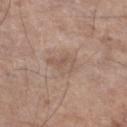{
  "biopsy_status": "not biopsied; imaged during a skin examination",
  "automated_metrics": {
    "cielab_L": 55,
    "cielab_a": 16,
    "cielab_b": 25,
    "vs_skin_darker_L": 7.0,
    "vs_skin_contrast_norm": 4.5,
    "border_irregularity_0_10": 4.0,
    "color_variation_0_10": 3.0,
    "peripheral_color_asymmetry": 1.0,
    "nevus_likeness_0_100": 0,
    "lesion_detection_confidence_0_100": 100
  },
  "image": {
    "source": "total-body photography crop",
    "field_of_view_mm": 15
  },
  "lesion_size": {
    "long_diameter_mm_approx": 3.5
  },
  "site": "leg",
  "patient": {
    "sex": "male",
    "age_approx": 80
  }
}